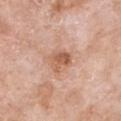Notes:
* workup · imaged on a skin check; not biopsied
* subject · female, aged approximately 75
* anatomic site · the chest
* TBP lesion metrics · a lesion area of about 6 mm² and two-axis asymmetry of about 0.3; a classifier nevus-likeness of about 35/100 and lesion-presence confidence of about 100/100
* acquisition · 15 mm crop, total-body photography
* tile lighting · white-light illumination